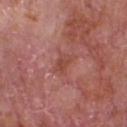  biopsy_status: not biopsied; imaged during a skin examination
  image:
    source: total-body photography crop
    field_of_view_mm: 15
  lighting: white-light
  site: chest
  patient:
    sex: male
    age_approx: 65